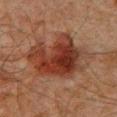  biopsy_status: not biopsied; imaged during a skin examination
  patient:
    sex: male
    age_approx: 60
  automated_metrics:
    eccentricity: 0.65
    shape_asymmetry: 0.35
    vs_skin_darker_L: 10.0
    vs_skin_contrast_norm: 9.5
    border_irregularity_0_10: 5.5
    color_variation_0_10: 6.0
    peripheral_color_asymmetry: 2.0
    nevus_likeness_0_100: 85
    lesion_detection_confidence_0_100: 100
  lesion_size:
    long_diameter_mm_approx: 8.0
  lighting: cross-polarized
  site: front of the torso
  image:
    source: total-body photography crop
    field_of_view_mm: 15